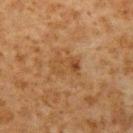Captured during whole-body skin photography for melanoma surveillance; the lesion was not biopsied. A close-up tile cropped from a whole-body skin photograph, about 15 mm across. A male patient in their 60s. From the arm. About 3 mm across. Imaged with cross-polarized lighting. The total-body-photography lesion software estimated a lesion color around L≈40 a*≈18 b*≈33 in CIELAB, a lesion–skin lightness drop of about 6, and a lesion-to-skin contrast of about 5.5 (normalized; higher = more distinct). The analysis additionally found border irregularity of about 3.5 on a 0–10 scale and peripheral color asymmetry of about 2.5. It also reported an automated nevus-likeness rating near 0 out of 100 and lesion-presence confidence of about 100/100.